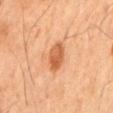Assessment: The lesion was tiled from a total-body skin photograph and was not biopsied. Acquisition and patient details: A 15 mm close-up extracted from a 3D total-body photography capture. On the mid back. A male patient aged 63 to 67.